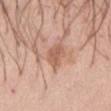The lesion was tiled from a total-body skin photograph and was not biopsied.
Located on the abdomen.
Imaged with white-light lighting.
The subject is a female aged 63 to 67.
The lesion's longest dimension is about 3 mm.
Cropped from a whole-body photographic skin survey; the tile spans about 15 mm.